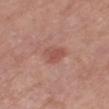  biopsy_status: not biopsied; imaged during a skin examination
  lesion_size:
    long_diameter_mm_approx: 3.0
  lighting: white-light
  patient:
    sex: male
    age_approx: 80
  image:
    source: total-body photography crop
    field_of_view_mm: 15
  automated_metrics:
    area_mm2_approx: 6.0
    eccentricity: 0.6
    shape_asymmetry: 0.3
    cielab_L: 52
    cielab_a: 25
    cielab_b: 26
    vs_skin_darker_L: 8.0
    vs_skin_contrast_norm: 6.0
    border_irregularity_0_10: 2.5
    color_variation_0_10: 2.5
    peripheral_color_asymmetry: 1.0
    lesion_detection_confidence_0_100: 100
  site: right thigh